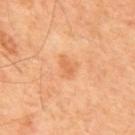* follow-up · imaged on a skin check; not biopsied
* image source · ~15 mm tile from a whole-body skin photo
* body site · the mid back
* subject · male, aged 63–67
* illumination · cross-polarized
* lesion diameter · ~3 mm (longest diameter)
* TBP lesion metrics · a lesion area of about 3.5 mm² and a shape eccentricity near 0.75; a color-variation rating of about 1/10 and a peripheral color-asymmetry measure near 0.5; a classifier nevus-likeness of about 0/100 and a lesion-detection confidence of about 100/100